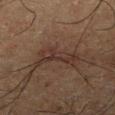Impression: The lesion was tiled from a total-body skin photograph and was not biopsied. Image and clinical context: A male patient, aged 63 to 67. About 5.5 mm across. Located on the right lower leg. Automated image analysis of the tile measured a lesion area of about 9 mm², an outline eccentricity of about 0.9 (0 = round, 1 = elongated), and a shape-asymmetry score of about 0.4 (0 = symmetric). And it measured border irregularity of about 6.5 on a 0–10 scale, a within-lesion color-variation index near 3/10, and a peripheral color-asymmetry measure near 1. The software also gave a classifier nevus-likeness of about 5/100 and lesion-presence confidence of about 65/100. A roughly 15 mm field-of-view crop from a total-body skin photograph.